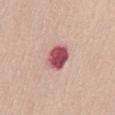No biopsy was performed on this lesion — it was imaged during a full skin examination and was not determined to be concerning. Automated tile analysis of the lesion measured a lesion–skin lightness drop of about 19 and a lesion-to-skin contrast of about 12.5 (normalized; higher = more distinct). The software also gave a detector confidence of about 100 out of 100 that the crop contains a lesion. About 3.5 mm across. The tile uses white-light illumination. Cropped from a total-body skin-imaging series; the visible field is about 15 mm. A female subject approximately 60 years of age. On the abdomen.